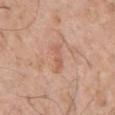Captured during whole-body skin photography for melanoma surveillance; the lesion was not biopsied.
The lesion is located on the left upper arm.
An algorithmic analysis of the crop reported a footprint of about 3.5 mm², an eccentricity of roughly 0.9, and a symmetry-axis asymmetry near 0.35. It also reported an average lesion color of about L≈59 a*≈23 b*≈31 (CIELAB), a lesion–skin lightness drop of about 7, and a lesion-to-skin contrast of about 5 (normalized; higher = more distinct). It also reported a peripheral color-asymmetry measure near 0. The software also gave an automated nevus-likeness rating near 0 out of 100.
The patient is a male aged 68–72.
A close-up tile cropped from a whole-body skin photograph, about 15 mm across.
About 3 mm across.